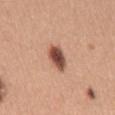Assessment: Part of a total-body skin-imaging series; this lesion was reviewed on a skin check and was not flagged for biopsy. Acquisition and patient details: Automated image analysis of the tile measured an eccentricity of roughly 0.8 and two-axis asymmetry of about 0.15. The software also gave a lesion color around L≈51 a*≈23 b*≈28 in CIELAB, roughly 17 lightness units darker than nearby skin, and a normalized border contrast of about 11.5. The analysis additionally found a border-irregularity rating of about 1.5/10, a within-lesion color-variation index near 6/10, and peripheral color asymmetry of about 1.5. And it measured an automated nevus-likeness rating near 100 out of 100. A female subject approximately 30 years of age. Cropped from a total-body skin-imaging series; the visible field is about 15 mm. From the mid back. Imaged with white-light lighting.